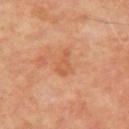The lesion was tiled from a total-body skin photograph and was not biopsied. A region of skin cropped from a whole-body photographic capture, roughly 15 mm wide. The subject is a male approximately 50 years of age. On the arm.